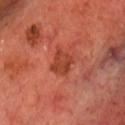Case summary:
• workup — catalogued during a skin exam; not biopsied
• location — the head or neck
• acquisition — ~15 mm crop, total-body skin-cancer survey
• subject — male, roughly 70 years of age